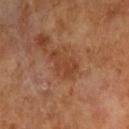A 15 mm close-up extracted from a 3D total-body photography capture. The lesion is located on the right upper arm. A male patient aged around 65.